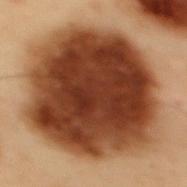Assessment:
No biopsy was performed on this lesion — it was imaged during a full skin examination and was not determined to be concerning.
Acquisition and patient details:
A male patient in their mid-50s. A 15 mm close-up extracted from a 3D total-body photography capture. The total-body-photography lesion software estimated a mean CIELAB color near L≈31 a*≈21 b*≈28 and a normalized border contrast of about 16.5. The software also gave a color-variation rating of about 6/10. Approximately 11 mm at its widest. Located on the mid back. Imaged with cross-polarized lighting.